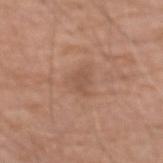notes — total-body-photography surveillance lesion; no biopsy | lighting — white-light | site — the left forearm | size — ≈2.5 mm | imaging modality — ~15 mm tile from a whole-body skin photo | TBP lesion metrics — a footprint of about 3.5 mm² and an outline eccentricity of about 0.7 (0 = round, 1 = elongated); an average lesion color of about L≈52 a*≈20 b*≈29 (CIELAB) and roughly 7 lightness units darker than nearby skin; a border-irregularity rating of about 3/10, a within-lesion color-variation index near 1/10, and peripheral color asymmetry of about 0.5 | subject — male, about 80 years old.